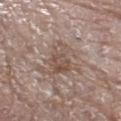The lesion was tiled from a total-body skin photograph and was not biopsied.